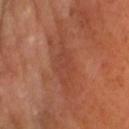notes=no biopsy performed (imaged during a skin exam)
image=~15 mm crop, total-body skin-cancer survey
site=the head or neck
patient=male, in their mid-60s
lighting=cross-polarized illumination
diameter=~5 mm (longest diameter)